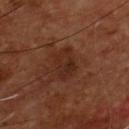This lesion was catalogued during total-body skin photography and was not selected for biopsy. A male patient aged 53–57. A close-up tile cropped from a whole-body skin photograph, about 15 mm across. Approximately 4 mm at its widest. From the chest. Captured under cross-polarized illumination.